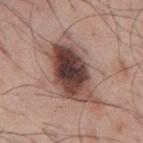Clinical impression:
Recorded during total-body skin imaging; not selected for excision or biopsy.
Context:
A region of skin cropped from a whole-body photographic capture, roughly 15 mm wide. Captured under white-light illumination. Located on the mid back. The patient is a male approximately 55 years of age.